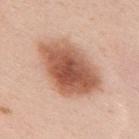workup: total-body-photography surveillance lesion; no biopsy | image: total-body-photography crop, ~15 mm field of view | location: the upper back | patient: female, aged approximately 30 | lighting: white-light illumination.